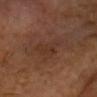| field | value |
|---|---|
| workup | catalogued during a skin exam; not biopsied |
| subject | male, aged 68–72 |
| body site | the head or neck |
| image source | total-body-photography crop, ~15 mm field of view |
| automated metrics | a border-irregularity rating of about 5.5/10 and a color-variation rating of about 2/10 |
| lighting | cross-polarized illumination |
| lesion diameter | ≈4.5 mm |